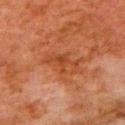Assessment: Captured during whole-body skin photography for melanoma surveillance; the lesion was not biopsied. Clinical summary: The subject is a male roughly 80 years of age. The lesion's longest dimension is about 4.5 mm. Cropped from a total-body skin-imaging series; the visible field is about 15 mm. Located on the left upper arm. Imaged with cross-polarized lighting.